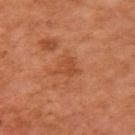<tbp_lesion>
  <biopsy_status>not biopsied; imaged during a skin examination</biopsy_status>
  <site>right upper arm</site>
  <image>
    <source>total-body photography crop</source>
    <field_of_view_mm>15</field_of_view_mm>
  </image>
  <patient>
    <sex>female</sex>
    <age_approx>60</age_approx>
  </patient>
  <lighting>cross-polarized</lighting>
</tbp_lesion>